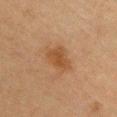Background: The subject is a female aged around 60. Located on the chest. This image is a 15 mm lesion crop taken from a total-body photograph. Approximately 4 mm at its widest. Captured under cross-polarized illumination.